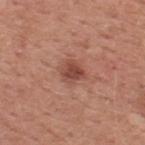workup=no biopsy performed (imaged during a skin exam) | imaging modality=~15 mm crop, total-body skin-cancer survey | subject=male, aged 48 to 52 | site=the upper back.